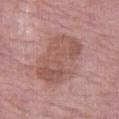Impression:
Imaged during a routine full-body skin examination; the lesion was not biopsied and no histopathology is available.
Clinical summary:
Automated tile analysis of the lesion measured a footprint of about 26 mm² and a symmetry-axis asymmetry near 0.2. It also reported a mean CIELAB color near L≈54 a*≈22 b*≈24, about 9 CIELAB-L* units darker than the surrounding skin, and a lesion-to-skin contrast of about 6.5 (normalized; higher = more distinct). The software also gave a nevus-likeness score of about 0/100 and a lesion-detection confidence of about 100/100. This is a white-light tile. A roughly 15 mm field-of-view crop from a total-body skin photograph. The patient is a female roughly 65 years of age. Longest diameter approximately 7 mm. From the leg.